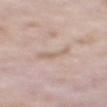Context: Approximately 3 mm at its widest. An algorithmic analysis of the crop reported a border-irregularity rating of about 5/10, a within-lesion color-variation index near 1/10, and radial color variation of about 0.5. On the mid back. Captured under white-light illumination. Cropped from a total-body skin-imaging series; the visible field is about 15 mm. A male subject approximately 60 years of age.